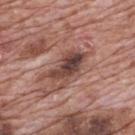This lesion was catalogued during total-body skin photography and was not selected for biopsy. A lesion tile, about 15 mm wide, cut from a 3D total-body photograph. From the back. A male patient aged around 70.